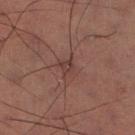Clinical impression: Captured during whole-body skin photography for melanoma surveillance; the lesion was not biopsied.